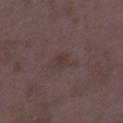{
  "biopsy_status": "not biopsied; imaged during a skin examination",
  "automated_metrics": {
    "area_mm2_approx": 4.5,
    "shape_asymmetry": 0.35,
    "border_irregularity_0_10": 4.0,
    "color_variation_0_10": 1.0,
    "peripheral_color_asymmetry": 0.5,
    "nevus_likeness_0_100": 0,
    "lesion_detection_confidence_0_100": 100
  },
  "image": {
    "source": "total-body photography crop",
    "field_of_view_mm": 15
  },
  "lesion_size": {
    "long_diameter_mm_approx": 3.5
  },
  "site": "right thigh",
  "lighting": "white-light",
  "patient": {
    "sex": "female",
    "age_approx": 35
  }
}